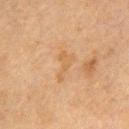notes = catalogued during a skin exam; not biopsied | image source = ~15 mm tile from a whole-body skin photo | illumination = cross-polarized illumination | lesion diameter = ≈4 mm | subject = female, aged 53 to 57 | body site = the chest | image-analysis metrics = an average lesion color of about L≈51 a*≈16 b*≈34 (CIELAB), about 5 CIELAB-L* units darker than the surrounding skin, and a normalized border contrast of about 5; a classifier nevus-likeness of about 0/100 and a lesion-detection confidence of about 100/100.